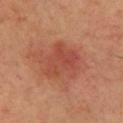Q: What is the anatomic site?
A: the chest
Q: Illumination type?
A: cross-polarized
Q: What did automated image analysis measure?
A: a footprint of about 12 mm², an outline eccentricity of about 0.65 (0 = round, 1 = elongated), and a symmetry-axis asymmetry near 0.35; a lesion color around L≈45 a*≈29 b*≈30 in CIELAB, a lesion–skin lightness drop of about 7, and a normalized lesion–skin contrast near 5.5; a nevus-likeness score of about 10/100
Q: What is the lesion's diameter?
A: ≈4.5 mm
Q: Who is the patient?
A: male, aged approximately 70
Q: What is the imaging modality?
A: ~15 mm tile from a whole-body skin photo